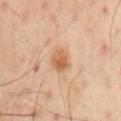  biopsy_status: not biopsied; imaged during a skin examination
  site: left arm
  patient:
    sex: male
    age_approx: 50
  automated_metrics:
    cielab_L: 60
    cielab_a: 22
    cielab_b: 37
    vs_skin_darker_L: 10.0
    vs_skin_contrast_norm: 7.5
    nevus_likeness_0_100: 90
    lesion_detection_confidence_0_100: 100
  image:
    source: total-body photography crop
    field_of_view_mm: 15